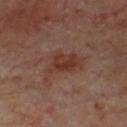Findings:
• workup · catalogued during a skin exam; not biopsied
• location · the front of the torso
• imaging modality · 15 mm crop, total-body photography
• size · ≈5.5 mm
• automated metrics · an average lesion color of about L≈34 a*≈19 b*≈25 (CIELAB) and roughly 7 lightness units darker than nearby skin; a nevus-likeness score of about 20/100 and lesion-presence confidence of about 100/100
• subject · male, roughly 70 years of age
• illumination · cross-polarized